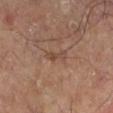size=about 3 mm
location=the left lower leg
imaging modality=~15 mm crop, total-body skin-cancer survey
tile lighting=cross-polarized illumination